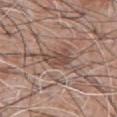Findings:
* follow-up · total-body-photography surveillance lesion; no biopsy
* subject · male, roughly 60 years of age
* image-analysis metrics · an area of roughly 6 mm², an eccentricity of roughly 0.8, and a shape-asymmetry score of about 0.3 (0 = symmetric); a mean CIELAB color near L≈47 a*≈18 b*≈24 and a normalized border contrast of about 7; a border-irregularity index near 4/10, a color-variation rating of about 3.5/10, and peripheral color asymmetry of about 1.5; an automated nevus-likeness rating near 0 out of 100
* image · ~15 mm tile from a whole-body skin photo
* body site · the chest
* illumination · white-light illumination